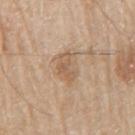Q: Was this lesion biopsied?
A: catalogued during a skin exam; not biopsied
Q: What is the lesion's diameter?
A: about 4 mm
Q: Illumination type?
A: white-light illumination
Q: What is the anatomic site?
A: the right upper arm
Q: How was this image acquired?
A: 15 mm crop, total-body photography
Q: What did automated image analysis measure?
A: a footprint of about 7.5 mm², an outline eccentricity of about 0.8 (0 = round, 1 = elongated), and a shape-asymmetry score of about 0.25 (0 = symmetric); a mean CIELAB color near L≈59 a*≈16 b*≈32 and a normalized lesion–skin contrast near 5.5; a nevus-likeness score of about 30/100
Q: What are the patient's age and sex?
A: male, roughly 80 years of age